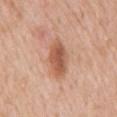This lesion was catalogued during total-body skin photography and was not selected for biopsy. The tile uses white-light illumination. Automated image analysis of the tile measured a mean CIELAB color near L≈57 a*≈23 b*≈33, a lesion–skin lightness drop of about 12, and a normalized lesion–skin contrast near 8. It also reported a color-variation rating of about 4.5/10 and radial color variation of about 1.5. A close-up tile cropped from a whole-body skin photograph, about 15 mm across. On the chest. A male subject, in their 60s. The recorded lesion diameter is about 5 mm.